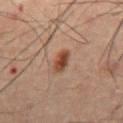follow-up: catalogued during a skin exam; not biopsied | site: the abdomen | subject: male, aged around 60 | imaging modality: 15 mm crop, total-body photography.